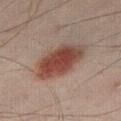Impression: Recorded during total-body skin imaging; not selected for excision or biopsy. Image and clinical context: Automated image analysis of the tile measured a lesion color around L≈39 a*≈19 b*≈23 in CIELAB and about 12 CIELAB-L* units darker than the surrounding skin. The software also gave a nevus-likeness score of about 100/100 and a detector confidence of about 100 out of 100 that the crop contains a lesion. A lesion tile, about 15 mm wide, cut from a 3D total-body photograph. This is a cross-polarized tile. The lesion is on the leg. A male patient roughly 40 years of age.